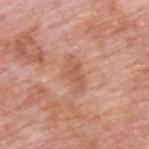follow-up: imaged on a skin check; not biopsied
location: the upper back
acquisition: total-body-photography crop, ~15 mm field of view
automated lesion analysis: a lesion area of about 7 mm² and an eccentricity of roughly 0.85
lesion diameter: ≈4 mm
patient: male, approximately 60 years of age
illumination: white-light